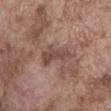Impression:
Part of a total-body skin-imaging series; this lesion was reviewed on a skin check and was not flagged for biopsy.
Context:
The total-body-photography lesion software estimated a lesion color around L≈47 a*≈19 b*≈22 in CIELAB, roughly 9 lightness units darker than nearby skin, and a normalized border contrast of about 7. And it measured a border-irregularity rating of about 3.5/10, a color-variation rating of about 3/10, and a peripheral color-asymmetry measure near 1. Longest diameter approximately 4.5 mm. On the abdomen. A male patient, aged 73–77. This is a white-light tile. A close-up tile cropped from a whole-body skin photograph, about 15 mm across.